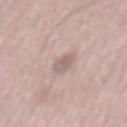Impression: The lesion was tiled from a total-body skin photograph and was not biopsied.